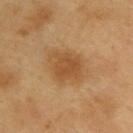Q: Was this lesion biopsied?
A: no biopsy performed (imaged during a skin exam)
Q: Patient demographics?
A: male, aged 58 to 62
Q: Lesion location?
A: the upper back
Q: Automated lesion metrics?
A: an area of roughly 14 mm² and an outline eccentricity of about 0.6 (0 = round, 1 = elongated); a border-irregularity rating of about 2/10, a within-lesion color-variation index near 2.5/10, and a peripheral color-asymmetry measure near 0.5
Q: Lesion size?
A: ≈5 mm
Q: How was the tile lit?
A: cross-polarized
Q: What kind of image is this?
A: 15 mm crop, total-body photography